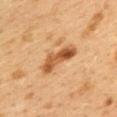Captured during whole-body skin photography for melanoma surveillance; the lesion was not biopsied.
Automated image analysis of the tile measured a lesion area of about 8 mm² and a shape eccentricity near 0.95. The software also gave a border-irregularity rating of about 4.5/10 and peripheral color asymmetry of about 2.5.
A 15 mm close-up tile from a total-body photography series done for melanoma screening.
From the upper back.
This is a cross-polarized tile.
A female subject, aged 38–42.